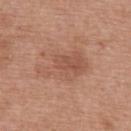Imaged during a routine full-body skin examination; the lesion was not biopsied and no histopathology is available.
This is a white-light tile.
A male subject aged around 55.
Cropped from a total-body skin-imaging series; the visible field is about 15 mm.
The recorded lesion diameter is about 6.5 mm.
From the upper back.